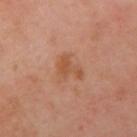This lesion was catalogued during total-body skin photography and was not selected for biopsy.
A female subject, in their mid- to late 50s.
The lesion's longest dimension is about 3 mm.
A close-up tile cropped from a whole-body skin photograph, about 15 mm across.
On the right upper arm.
Captured under cross-polarized illumination.